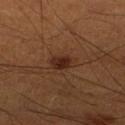The lesion was tiled from a total-body skin photograph and was not biopsied. Automated tile analysis of the lesion measured an eccentricity of roughly 0.75 and two-axis asymmetry of about 0.25. It also reported a mean CIELAB color near L≈27 a*≈20 b*≈27, about 9 CIELAB-L* units darker than the surrounding skin, and a lesion-to-skin contrast of about 9 (normalized; higher = more distinct). It also reported radial color variation of about 1. The software also gave an automated nevus-likeness rating near 100 out of 100 and a detector confidence of about 100 out of 100 that the crop contains a lesion. A region of skin cropped from a whole-body photographic capture, roughly 15 mm wide. From the right lower leg. Approximately 3 mm at its widest. The patient is a male roughly 50 years of age.